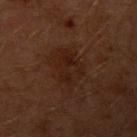Part of a total-body skin-imaging series; this lesion was reviewed on a skin check and was not flagged for biopsy. Captured under cross-polarized illumination. From the left upper arm. A male subject, aged approximately 60. Cropped from a total-body skin-imaging series; the visible field is about 15 mm. Longest diameter approximately 5 mm.